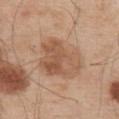Findings:
• follow-up: imaged on a skin check; not biopsied
• patient: male, aged approximately 55
• site: the upper back
• tile lighting: white-light
• size: ~6.5 mm (longest diameter)
• image source: 15 mm crop, total-body photography